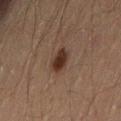Acquisition and patient details:
A 15 mm crop from a total-body photograph taken for skin-cancer surveillance. Captured under cross-polarized illumination. A male subject, roughly 60 years of age. The lesion is located on the leg.